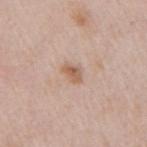follow-up: imaged on a skin check; not biopsied
imaging modality: 15 mm crop, total-body photography
illumination: white-light illumination
body site: the right upper arm
patient: female, approximately 40 years of age
diameter: about 2.5 mm